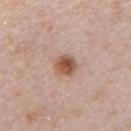{"biopsy_status": "not biopsied; imaged during a skin examination", "site": "chest", "patient": {"sex": "male", "age_approx": 40}, "image": {"source": "total-body photography crop", "field_of_view_mm": 15}, "lighting": "white-light", "automated_metrics": {"eccentricity": 0.4, "shape_asymmetry": 0.2, "color_variation_0_10": 5.5}, "lesion_size": {"long_diameter_mm_approx": 3.0}}